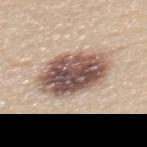Acquisition and patient details: A 15 mm close-up extracted from a 3D total-body photography capture. From the upper back. A female subject aged 23–27.